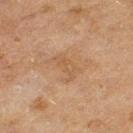notes=no biopsy performed (imaged during a skin exam)
subject=female, roughly 60 years of age
location=the left thigh
lighting=cross-polarized illumination
TBP lesion metrics=a lesion–skin lightness drop of about 5
image=~15 mm crop, total-body skin-cancer survey